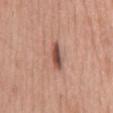{"biopsy_status": "not biopsied; imaged during a skin examination", "site": "mid back", "image": {"source": "total-body photography crop", "field_of_view_mm": 15}, "patient": {"sex": "male", "age_approx": 60}}